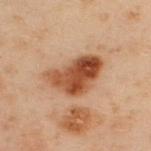The lesion was photographed on a routine skin check and not biopsied; there is no pathology result. Located on the upper back. A close-up tile cropped from a whole-body skin photograph, about 15 mm across. A male patient, aged 48–52.